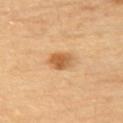• notes: no biopsy performed (imaged during a skin exam)
• body site: the upper back
• automated metrics: an area of roughly 5.5 mm² and an eccentricity of roughly 0.75; a lesion color around L≈57 a*≈22 b*≈41 in CIELAB and a normalized border contrast of about 8.5
• image source: ~15 mm tile from a whole-body skin photo
• tile lighting: cross-polarized
• patient: male, approximately 85 years of age or older